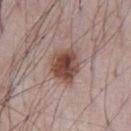Assessment:
The lesion was tiled from a total-body skin photograph and was not biopsied.
Background:
On the chest. A male subject in their mid- to late 60s. This image is a 15 mm lesion crop taken from a total-body photograph.